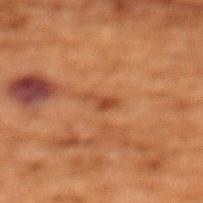Clinical impression: No biopsy was performed on this lesion — it was imaged during a full skin examination and was not determined to be concerning. Image and clinical context: A female subject, aged around 80. From the chest. A region of skin cropped from a whole-body photographic capture, roughly 15 mm wide. The tile uses cross-polarized illumination. About 3 mm across.